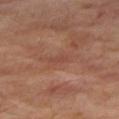Clinical impression:
Part of a total-body skin-imaging series; this lesion was reviewed on a skin check and was not flagged for biopsy.
Clinical summary:
On the right thigh. Cropped from a whole-body photographic skin survey; the tile spans about 15 mm. The lesion-visualizer software estimated a mean CIELAB color near L≈44 a*≈23 b*≈27, about 6 CIELAB-L* units darker than the surrounding skin, and a normalized lesion–skin contrast near 4.5. It also reported a border-irregularity rating of about 4/10, a color-variation rating of about 0/10, and a peripheral color-asymmetry measure near 0. The patient is a male approximately 70 years of age.